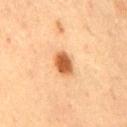notes=catalogued during a skin exam; not biopsied
location=the mid back
subject=male, aged 63–67
image source=~15 mm tile from a whole-body skin photo